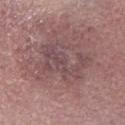notes = no biopsy performed (imaged during a skin exam)
illumination = white-light
subject = female, aged approximately 60
image source = 15 mm crop, total-body photography
body site = the abdomen